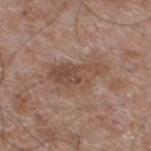Part of a total-body skin-imaging series; this lesion was reviewed on a skin check and was not flagged for biopsy.
Located on the left lower leg.
A region of skin cropped from a whole-body photographic capture, roughly 15 mm wide.
A male patient about 60 years old.
The tile uses white-light illumination.
The lesion-visualizer software estimated a lesion color around L≈48 a*≈18 b*≈26 in CIELAB, roughly 8 lightness units darker than nearby skin, and a normalized lesion–skin contrast near 6.5. It also reported an automated nevus-likeness rating near 0 out of 100 and lesion-presence confidence of about 100/100.
Measured at roughly 6.5 mm in maximum diameter.